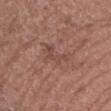Clinical impression:
The lesion was photographed on a routine skin check and not biopsied; there is no pathology result.
Image and clinical context:
A male patient aged around 65. The lesion is located on the left forearm. A close-up tile cropped from a whole-body skin photograph, about 15 mm across. The tile uses white-light illumination.